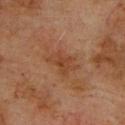No biopsy was performed on this lesion — it was imaged during a full skin examination and was not determined to be concerning.
A male subject, roughly 75 years of age.
The lesion is on the upper back.
Cropped from a total-body skin-imaging series; the visible field is about 15 mm.